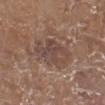Q: Is there a histopathology result?
A: no biopsy performed (imaged during a skin exam)
Q: Automated lesion metrics?
A: an area of roughly 13 mm², an eccentricity of roughly 0.7, and two-axis asymmetry of about 0.2; a lesion–skin lightness drop of about 8
Q: Who is the patient?
A: female, in their mid-70s
Q: Lesion location?
A: the left lower leg
Q: How was the tile lit?
A: white-light
Q: What is the lesion's diameter?
A: ≈5 mm
Q: What kind of image is this?
A: ~15 mm crop, total-body skin-cancer survey